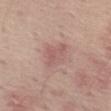Captured during whole-body skin photography for melanoma surveillance; the lesion was not biopsied. A male patient aged 63 to 67. The tile uses white-light illumination. The lesion is on the leg. A lesion tile, about 15 mm wide, cut from a 3D total-body photograph.